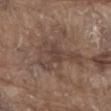Impression: The lesion was tiled from a total-body skin photograph and was not biopsied. Acquisition and patient details: The patient is a male aged 78 to 82. Captured under white-light illumination. Measured at roughly 6 mm in maximum diameter. A 15 mm close-up tile from a total-body photography series done for melanoma screening. The lesion-visualizer software estimated a lesion area of about 14 mm², an outline eccentricity of about 0.85 (0 = round, 1 = elongated), and a shape-asymmetry score of about 0.6 (0 = symmetric). And it measured a lesion color around L≈42 a*≈16 b*≈23 in CIELAB and a lesion-to-skin contrast of about 6.5 (normalized; higher = more distinct). The lesion is located on the abdomen.